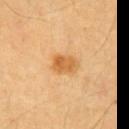Clinical impression:
The lesion was photographed on a routine skin check and not biopsied; there is no pathology result.
Clinical summary:
The subject is a male approximately 60 years of age. The lesion is on the chest. Measured at roughly 3 mm in maximum diameter. The lesion-visualizer software estimated an average lesion color of about L≈59 a*≈22 b*≈44 (CIELAB), roughly 10 lightness units darker than nearby skin, and a normalized lesion–skin contrast near 7.5. And it measured lesion-presence confidence of about 100/100. The tile uses cross-polarized illumination. A close-up tile cropped from a whole-body skin photograph, about 15 mm across.